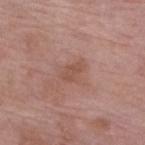Assessment: Part of a total-body skin-imaging series; this lesion was reviewed on a skin check and was not flagged for biopsy. Acquisition and patient details: A female patient, aged 68–72. About 2.5 mm across. The tile uses white-light illumination. From the right lower leg. A lesion tile, about 15 mm wide, cut from a 3D total-body photograph.